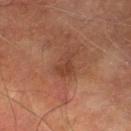The lesion was tiled from a total-body skin photograph and was not biopsied. The lesion is located on the left thigh. A male patient, aged around 75. A 15 mm crop from a total-body photograph taken for skin-cancer surveillance.